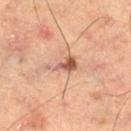Findings:
• biopsy status · total-body-photography surveillance lesion; no biopsy
• acquisition · total-body-photography crop, ~15 mm field of view
• illumination · cross-polarized
• anatomic site · the leg
• patient · male, in their mid-60s
• diameter · ~4 mm (longest diameter)